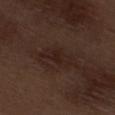Clinical summary:
Captured under white-light illumination. A close-up tile cropped from a whole-body skin photograph, about 15 mm across. About 4 mm across. From the right thigh. The lesion-visualizer software estimated a footprint of about 5.5 mm² and an outline eccentricity of about 0.9 (0 = round, 1 = elongated). And it measured border irregularity of about 4.5 on a 0–10 scale and internal color variation of about 3.5 on a 0–10 scale. A male subject approximately 70 years of age.